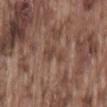The lesion was tiled from a total-body skin photograph and was not biopsied.
Cropped from a whole-body photographic skin survey; the tile spans about 15 mm.
The lesion is located on the lower back.
Captured under white-light illumination.
A male patient in their mid-70s.